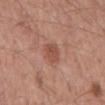Notes:
– follow-up · total-body-photography surveillance lesion; no biopsy
– subject · male, in their mid- to late 50s
– body site · the mid back
– image source · ~15 mm tile from a whole-body skin photo
– TBP lesion metrics · a mean CIELAB color near L≈50 a*≈24 b*≈28 and a lesion–skin lightness drop of about 9
– lighting · white-light illumination
– lesion size · ~3 mm (longest diameter)